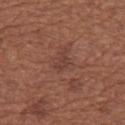| key | value |
|---|---|
| patient | female, in their mid-50s |
| size | ≈2.5 mm |
| lighting | white-light |
| site | the left thigh |
| automated lesion analysis | a shape-asymmetry score of about 0.45 (0 = symmetric); a normalized lesion–skin contrast near 5.5; a border-irregularity index near 4/10 and a within-lesion color-variation index near 1.5/10 |
| imaging modality | 15 mm crop, total-body photography |